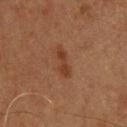Case summary:
* workup: imaged on a skin check; not biopsied
* diameter: about 4 mm
* patient: male, roughly 60 years of age
* illumination: cross-polarized
* automated metrics: an average lesion color of about L≈34 a*≈21 b*≈29 (CIELAB), about 7 CIELAB-L* units darker than the surrounding skin, and a normalized lesion–skin contrast near 7; a border-irregularity index near 4.5/10, internal color variation of about 1 on a 0–10 scale, and a peripheral color-asymmetry measure near 0; a nevus-likeness score of about 5/100 and a detector confidence of about 100 out of 100 that the crop contains a lesion
* image: ~15 mm crop, total-body skin-cancer survey